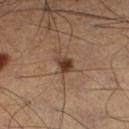Acquisition and patient details:
An algorithmic analysis of the crop reported a lesion area of about 4 mm² and a shape-asymmetry score of about 0.3 (0 = symmetric). And it measured a border-irregularity rating of about 3/10, internal color variation of about 4.5 on a 0–10 scale, and radial color variation of about 1.5. The patient is a male aged 48 to 52. Measured at roughly 3 mm in maximum diameter. This image is a 15 mm lesion crop taken from a total-body photograph. Captured under cross-polarized illumination. The lesion is on the left leg.